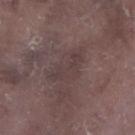Imaged during a routine full-body skin examination; the lesion was not biopsied and no histopathology is available. Imaged with white-light lighting. The patient is a male in their mid- to late 70s. The recorded lesion diameter is about 5.5 mm. The lesion-visualizer software estimated a lesion area of about 9 mm² and a shape eccentricity near 0.9. The analysis additionally found about 5 CIELAB-L* units darker than the surrounding skin. A 15 mm crop from a total-body photograph taken for skin-cancer surveillance. From the leg.